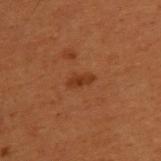Findings:
- biopsy status · imaged on a skin check; not biopsied
- subject · male, aged around 50
- site · the upper back
- image source · 15 mm crop, total-body photography
- lighting · cross-polarized
- lesion diameter · ≈2.5 mm